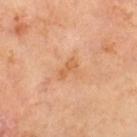Assessment: Recorded during total-body skin imaging; not selected for excision or biopsy.